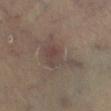Assessment: Recorded during total-body skin imaging; not selected for excision or biopsy. Background: A lesion tile, about 15 mm wide, cut from a 3D total-body photograph. From the left lower leg. A male patient, about 60 years old.